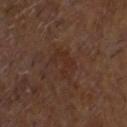Imaged during a routine full-body skin examination; the lesion was not biopsied and no histopathology is available. A male subject aged around 60. A roughly 15 mm field-of-view crop from a total-body skin photograph. Located on the head or neck. The lesion's longest dimension is about 3.5 mm. Captured under cross-polarized illumination.